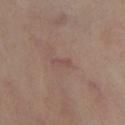Cropped from a total-body skin-imaging series; the visible field is about 15 mm. A female subject about 50 years old. Imaged with cross-polarized lighting. On the left thigh.